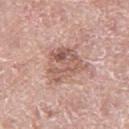{"biopsy_status": "not biopsied; imaged during a skin examination"}